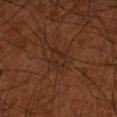Background:
The lesion is on the left arm. This is a cross-polarized tile. Longest diameter approximately 2.5 mm. A region of skin cropped from a whole-body photographic capture, roughly 15 mm wide. A male patient roughly 50 years of age.